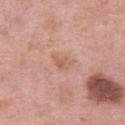The lesion was tiled from a total-body skin photograph and was not biopsied. The lesion is on the left thigh. Cropped from a whole-body photographic skin survey; the tile spans about 15 mm. A female subject, in their 40s. The lesion-visualizer software estimated a border-irregularity rating of about 4/10, a within-lesion color-variation index near 2.5/10, and radial color variation of about 1.